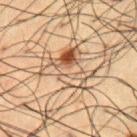Part of a total-body skin-imaging series; this lesion was reviewed on a skin check and was not flagged for biopsy.
Longest diameter approximately 6.5 mm.
Cropped from a total-body skin-imaging series; the visible field is about 15 mm.
The patient is a male approximately 60 years of age.
On the chest.